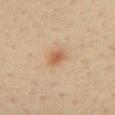{"biopsy_status": "not biopsied; imaged during a skin examination", "image": {"source": "total-body photography crop", "field_of_view_mm": 15}, "site": "chest", "automated_metrics": {"vs_skin_darker_L": 8.0, "border_irregularity_0_10": 2.0, "color_variation_0_10": 2.5, "peripheral_color_asymmetry": 1.0}, "lesion_size": {"long_diameter_mm_approx": 2.5}, "patient": {"sex": "female", "age_approx": 40}}